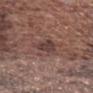The lesion was tiled from a total-body skin photograph and was not biopsied.